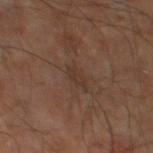The subject is a male aged approximately 60.
A region of skin cropped from a whole-body photographic capture, roughly 15 mm wide.
The lesion-visualizer software estimated a lesion area of about 2.5 mm², a shape eccentricity near 0.85, and two-axis asymmetry of about 0.25. The software also gave a lesion color around L≈32 a*≈15 b*≈24 in CIELAB and a normalized lesion–skin contrast near 5. And it measured a classifier nevus-likeness of about 0/100 and a detector confidence of about 100 out of 100 that the crop contains a lesion.
The recorded lesion diameter is about 2.5 mm.
Imaged with cross-polarized lighting.
Located on the right leg.